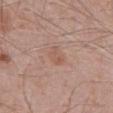anatomic site: the abdomen; lesion size: about 2.5 mm; patient: male, roughly 70 years of age; imaging modality: 15 mm crop, total-body photography.